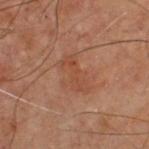This lesion was catalogued during total-body skin photography and was not selected for biopsy. An algorithmic analysis of the crop reported a lesion area of about 5 mm², an eccentricity of roughly 0.95, and two-axis asymmetry of about 0.45. This is a cross-polarized tile. A roughly 15 mm field-of-view crop from a total-body skin photograph. The lesion is located on the chest. The recorded lesion diameter is about 4 mm. The patient is a male aged around 70.